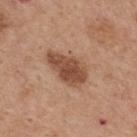Imaged during a routine full-body skin examination; the lesion was not biopsied and no histopathology is available.
From the upper back.
A male patient aged around 65.
Cropped from a whole-body photographic skin survey; the tile spans about 15 mm.
The lesion's longest dimension is about 5.5 mm.
The total-body-photography lesion software estimated a lesion color around L≈49 a*≈21 b*≈31 in CIELAB, roughly 13 lightness units darker than nearby skin, and a normalized border contrast of about 9. And it measured border irregularity of about 3 on a 0–10 scale, a color-variation rating of about 3.5/10, and radial color variation of about 1. The analysis additionally found a classifier nevus-likeness of about 75/100 and a detector confidence of about 100 out of 100 that the crop contains a lesion.